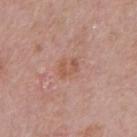Impression: Imaged during a routine full-body skin examination; the lesion was not biopsied and no histopathology is available. Context: Imaged with white-light lighting. A male subject, aged 68 to 72. Cropped from a total-body skin-imaging series; the visible field is about 15 mm. The lesion-visualizer software estimated an average lesion color of about L≈55 a*≈22 b*≈29 (CIELAB), roughly 7 lightness units darker than nearby skin, and a normalized border contrast of about 5.5. The software also gave border irregularity of about 3.5 on a 0–10 scale, internal color variation of about 2 on a 0–10 scale, and radial color variation of about 0.5. On the front of the torso.